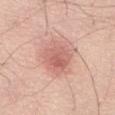Assessment:
This lesion was catalogued during total-body skin photography and was not selected for biopsy.
Context:
The lesion-visualizer software estimated an area of roughly 14 mm², an eccentricity of roughly 0.65, and a symmetry-axis asymmetry near 0.15. And it measured a border-irregularity index near 2/10, a color-variation rating of about 4.5/10, and radial color variation of about 1.5. And it measured a detector confidence of about 100 out of 100 that the crop contains a lesion. This is a white-light tile. Located on the abdomen. Measured at roughly 5 mm in maximum diameter. A 15 mm close-up tile from a total-body photography series done for melanoma screening. The subject is a male aged 68–72.